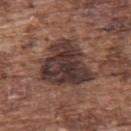The lesion was photographed on a routine skin check and not biopsied; there is no pathology result. On the upper back. This image is a 15 mm lesion crop taken from a total-body photograph. The patient is a male approximately 75 years of age.